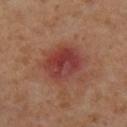biopsy_status: not biopsied; imaged during a skin examination
site: leg
automated_metrics:
  cielab_L: 41
  cielab_a: 28
  cielab_b: 26
  vs_skin_darker_L: 10.0
  vs_skin_contrast_norm: 8.5
  peripheral_color_asymmetry: 2.5
  nevus_likeness_0_100: 0
  lesion_detection_confidence_0_100: 100
image:
  source: total-body photography crop
  field_of_view_mm: 15
lighting: cross-polarized
lesion_size:
  long_diameter_mm_approx: 4.5
patient:
  sex: female
  age_approx: 55